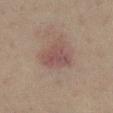| feature | finding |
|---|---|
| workup | imaged on a skin check; not biopsied |
| image source | ~15 mm tile from a whole-body skin photo |
| body site | the left lower leg |
| automated metrics | a border-irregularity rating of about 3.5/10 and a color-variation rating of about 2.5/10; a classifier nevus-likeness of about 20/100 and lesion-presence confidence of about 100/100 |
| tile lighting | cross-polarized |
| subject | female, aged 38–42 |